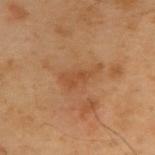Notes:
– follow-up · catalogued during a skin exam; not biopsied
– subject · male, about 55 years old
– site · the upper back
– acquisition · ~15 mm crop, total-body skin-cancer survey
– size · about 4.5 mm